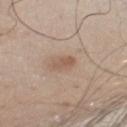Case summary:
* notes · no biopsy performed (imaged during a skin exam)
* subject · male, about 80 years old
* location · the chest
* imaging modality · 15 mm crop, total-body photography
* image-analysis metrics · a mean CIELAB color near L≈56 a*≈16 b*≈29, roughly 8 lightness units darker than nearby skin, and a lesion-to-skin contrast of about 6 (normalized; higher = more distinct); a classifier nevus-likeness of about 10/100 and a detector confidence of about 100 out of 100 that the crop contains a lesion